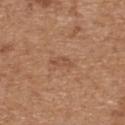| key | value |
|---|---|
| follow-up | imaged on a skin check; not biopsied |
| imaging modality | 15 mm crop, total-body photography |
| patient | male, in their 70s |
| site | the upper back |